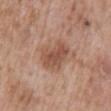Imaged during a routine full-body skin examination; the lesion was not biopsied and no histopathology is available.
A male subject, aged approximately 60.
A close-up tile cropped from a whole-body skin photograph, about 15 mm across.
Located on the abdomen.
Automated tile analysis of the lesion measured a shape eccentricity near 0.55. The analysis additionally found a mean CIELAB color near L≈52 a*≈21 b*≈29 and a normalized border contrast of about 7. It also reported border irregularity of about 2.5 on a 0–10 scale and internal color variation of about 3.5 on a 0–10 scale. The software also gave an automated nevus-likeness rating near 15 out of 100 and lesion-presence confidence of about 100/100.
Captured under white-light illumination.